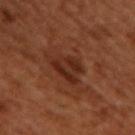Findings:
– follow-up · catalogued during a skin exam; not biopsied
– subject · male, aged 48–52
– location · the upper back
– imaging modality · 15 mm crop, total-body photography